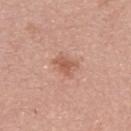follow-up: catalogued during a skin exam; not biopsied
patient: female, aged 53 to 57
image: total-body-photography crop, ~15 mm field of view
site: the arm